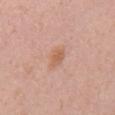* follow-up · imaged on a skin check; not biopsied
* image · ~15 mm crop, total-body skin-cancer survey
* lesion diameter · about 2.5 mm
* patient · male, roughly 55 years of age
* site · the chest